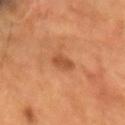<lesion>
  <biopsy_status>not biopsied; imaged during a skin examination</biopsy_status>
  <lighting>cross-polarized</lighting>
  <lesion_size>
    <long_diameter_mm_approx>3.0</long_diameter_mm_approx>
  </lesion_size>
  <patient>
    <sex>male</sex>
    <age_approx>55</age_approx>
  </patient>
  <image>
    <source>total-body photography crop</source>
    <field_of_view_mm>15</field_of_view_mm>
  </image>
  <site>head or neck</site>
</lesion>